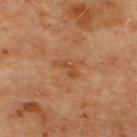Acquisition and patient details: Captured under cross-polarized illumination. The lesion is located on the upper back. A male subject aged 63–67. An algorithmic analysis of the crop reported a shape eccentricity near 0.9 and a symmetry-axis asymmetry near 0.5. And it measured an average lesion color of about L≈47 a*≈25 b*≈37 (CIELAB), about 7 CIELAB-L* units darker than the surrounding skin, and a normalized lesion–skin contrast near 6. The software also gave border irregularity of about 6.5 on a 0–10 scale. And it measured a nevus-likeness score of about 0/100 and lesion-presence confidence of about 100/100. Cropped from a whole-body photographic skin survey; the tile spans about 15 mm. Measured at roughly 2.5 mm in maximum diameter.